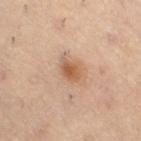Q: Is there a histopathology result?
A: imaged on a skin check; not biopsied
Q: Lesion location?
A: the right thigh
Q: What is the lesion's diameter?
A: ~3 mm (longest diameter)
Q: Patient demographics?
A: male, roughly 55 years of age
Q: What kind of image is this?
A: ~15 mm crop, total-body skin-cancer survey